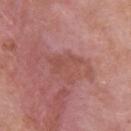<case>
  <patient>
    <sex>male</sex>
    <age_approx>40</age_approx>
  </patient>
  <site>upper back</site>
  <image>
    <source>total-body photography crop</source>
    <field_of_view_mm>15</field_of_view_mm>
  </image>
  <lighting>white-light</lighting>
  <lesion_size>
    <long_diameter_mm_approx>4.5</long_diameter_mm_approx>
  </lesion_size>
</case>